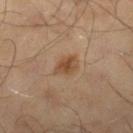notes=catalogued during a skin exam; not biopsied | lesion size=about 2.5 mm | acquisition=~15 mm tile from a whole-body skin photo | subject=male, aged approximately 65 | body site=the leg | illumination=cross-polarized.